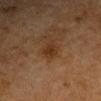<tbp_lesion>
  <biopsy_status>not biopsied; imaged during a skin examination</biopsy_status>
  <automated_metrics>
    <cielab_L>28</cielab_L>
    <cielab_a>18</cielab_a>
    <cielab_b>28</cielab_b>
    <vs_skin_darker_L>6.0</vs_skin_darker_L>
  </automated_metrics>
  <lesion_size>
    <long_diameter_mm_approx>3.0</long_diameter_mm_approx>
  </lesion_size>
  <patient>
    <sex>male</sex>
    <age_approx>65</age_approx>
  </patient>
  <image>
    <source>total-body photography crop</source>
    <field_of_view_mm>15</field_of_view_mm>
  </image>
  <site>right upper arm</site>
</tbp_lesion>